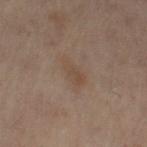This lesion was catalogued during total-body skin photography and was not selected for biopsy.
This is a cross-polarized tile.
The lesion-visualizer software estimated a symmetry-axis asymmetry near 0.35. And it measured lesion-presence confidence of about 100/100.
Longest diameter approximately 4.5 mm.
The subject is a female in their mid-50s.
The lesion is on the abdomen.
This image is a 15 mm lesion crop taken from a total-body photograph.